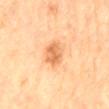- body site — the mid back
- diameter — about 4 mm
- image source — 15 mm crop, total-body photography
- lighting — cross-polarized
- subject — male, aged approximately 85 or older